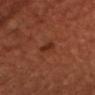The lesion was photographed on a routine skin check and not biopsied; there is no pathology result. A region of skin cropped from a whole-body photographic capture, roughly 15 mm wide. This is a cross-polarized tile. Approximately 3 mm at its widest. The subject is a female approximately 50 years of age.